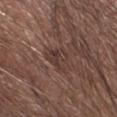follow-up: no biopsy performed (imaged during a skin exam)
site: the right forearm
automated metrics: a footprint of about 6.5 mm² and a shape eccentricity near 0.7; a classifier nevus-likeness of about 0/100 and lesion-presence confidence of about 60/100
image: ~15 mm tile from a whole-body skin photo
size: about 3.5 mm
patient: male, roughly 65 years of age
tile lighting: white-light